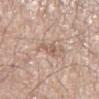Imaged during a routine full-body skin examination; the lesion was not biopsied and no histopathology is available.